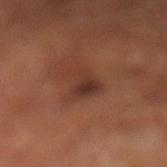Clinical impression:
This lesion was catalogued during total-body skin photography and was not selected for biopsy.
Background:
A region of skin cropped from a whole-body photographic capture, roughly 15 mm wide. About 4 mm across. From the left lower leg. A male patient, about 70 years old. The tile uses cross-polarized illumination.